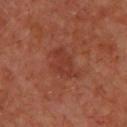Captured during whole-body skin photography for melanoma surveillance; the lesion was not biopsied.
The total-body-photography lesion software estimated a color-variation rating of about 2/10 and radial color variation of about 1.
Cropped from a whole-body photographic skin survey; the tile spans about 15 mm.
The lesion is on the upper back.
A male subject aged 68 to 72.
This is a cross-polarized tile.
Measured at roughly 4.5 mm in maximum diameter.